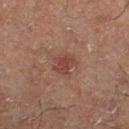Assessment: No biopsy was performed on this lesion — it was imaged during a full skin examination and was not determined to be concerning.